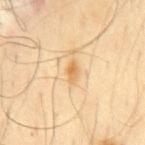workup — catalogued during a skin exam; not biopsied
patient — male, roughly 65 years of age
site — the mid back
automated lesion analysis — a lesion area of about 3 mm²; an average lesion color of about L≈66 a*≈17 b*≈42 (CIELAB), about 10 CIELAB-L* units darker than the surrounding skin, and a lesion-to-skin contrast of about 7 (normalized; higher = more distinct)
image — ~15 mm tile from a whole-body skin photo
lesion diameter — ~2.5 mm (longest diameter)
lighting — cross-polarized illumination